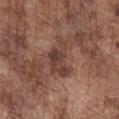{
  "biopsy_status": "not biopsied; imaged during a skin examination",
  "lighting": "white-light",
  "patient": {
    "sex": "male",
    "age_approx": 75
  },
  "site": "chest",
  "image": {
    "source": "total-body photography crop",
    "field_of_view_mm": 15
  },
  "lesion_size": {
    "long_diameter_mm_approx": 5.5
  }
}